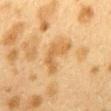| key | value |
|---|---|
| lighting | cross-polarized illumination |
| patient | female, about 40 years old |
| automated metrics | a lesion area of about 9 mm² and a symmetry-axis asymmetry near 0.5; a mean CIELAB color near L≈53 a*≈16 b*≈38, about 8 CIELAB-L* units darker than the surrounding skin, and a lesion-to-skin contrast of about 6.5 (normalized; higher = more distinct) |
| body site | the mid back |
| image | ~15 mm tile from a whole-body skin photo |